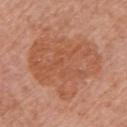Assessment:
Imaged during a routine full-body skin examination; the lesion was not biopsied and no histopathology is available.
Image and clinical context:
The lesion-visualizer software estimated a shape eccentricity near 0.6 and a shape-asymmetry score of about 0.3 (0 = symmetric). It also reported a mean CIELAB color near L≈54 a*≈25 b*≈34 and a normalized lesion–skin contrast near 6. A 15 mm close-up extracted from a 3D total-body photography capture. The patient is a female in their mid-50s. Approximately 8 mm at its widest. This is a white-light tile. The lesion is located on the left upper arm.